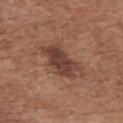The lesion was photographed on a routine skin check and not biopsied; there is no pathology result.
Imaged with white-light lighting.
The subject is a female roughly 75 years of age.
A 15 mm close-up extracted from a 3D total-body photography capture.
The lesion is on the upper back.